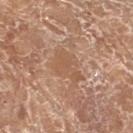notes = total-body-photography surveillance lesion; no biopsy
patient = female, aged approximately 75
body site = the left lower leg
acquisition = total-body-photography crop, ~15 mm field of view